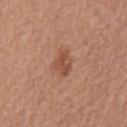Impression:
The lesion was photographed on a routine skin check and not biopsied; there is no pathology result.
Background:
This is a white-light tile. The lesion's longest dimension is about 3 mm. The lesion is located on the mid back. The lesion-visualizer software estimated a footprint of about 5 mm². It also reported a border-irregularity index near 3.5/10, internal color variation of about 2 on a 0–10 scale, and a peripheral color-asymmetry measure near 0.5. And it measured a classifier nevus-likeness of about 40/100 and lesion-presence confidence of about 100/100. A 15 mm close-up tile from a total-body photography series done for melanoma screening. The subject is a male in their mid-60s.